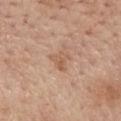Imaged during a routine full-body skin examination; the lesion was not biopsied and no histopathology is available.
Located on the mid back.
A female subject, aged around 65.
A 15 mm close-up extracted from a 3D total-body photography capture.